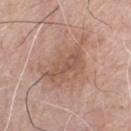Clinical impression: Recorded during total-body skin imaging; not selected for excision or biopsy. Clinical summary: Approximately 7 mm at its widest. This image is a 15 mm lesion crop taken from a total-body photograph. Located on the chest. Automated image analysis of the tile measured a lesion area of about 21 mm², a shape eccentricity near 0.75, and a symmetry-axis asymmetry near 0.35. The analysis additionally found a border-irregularity index near 5/10 and peripheral color asymmetry of about 1.5. The analysis additionally found an automated nevus-likeness rating near 0 out of 100 and a lesion-detection confidence of about 100/100. The subject is a male aged 78 to 82. The tile uses white-light illumination.